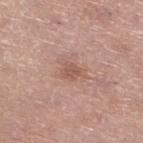The lesion is located on the left lower leg.
The patient is a female roughly 65 years of age.
A region of skin cropped from a whole-body photographic capture, roughly 15 mm wide.